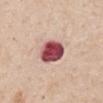Imaged during a routine full-body skin examination; the lesion was not biopsied and no histopathology is available. The patient is a male roughly 60 years of age. From the abdomen. The total-body-photography lesion software estimated an area of roughly 10 mm², an eccentricity of roughly 0.65, and a shape-asymmetry score of about 0.1 (0 = symmetric). And it measured an average lesion color of about L≈50 a*≈32 b*≈20 (CIELAB), a lesion–skin lightness drop of about 24, and a normalized lesion–skin contrast near 15.5. It also reported a border-irregularity rating of about 1.5/10, internal color variation of about 7 on a 0–10 scale, and peripheral color asymmetry of about 2. A lesion tile, about 15 mm wide, cut from a 3D total-body photograph. About 4 mm across. This is a white-light tile.